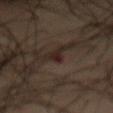This lesion was catalogued during total-body skin photography and was not selected for biopsy.
This is a cross-polarized tile.
On the abdomen.
The recorded lesion diameter is about 2.5 mm.
Automated image analysis of the tile measured an area of roughly 2 mm² and a symmetry-axis asymmetry near 0.3. The analysis additionally found an automated nevus-likeness rating near 0 out of 100 and a lesion-detection confidence of about 70/100.
A 15 mm close-up extracted from a 3D total-body photography capture.
A male patient about 55 years old.